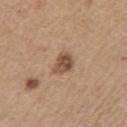<case>
<lighting>white-light</lighting>
<site>left upper arm</site>
<image>
  <source>total-body photography crop</source>
  <field_of_view_mm>15</field_of_view_mm>
</image>
<automated_metrics>
  <area_mm2_approx>5.5</area_mm2_approx>
  <shape_asymmetry>0.3</shape_asymmetry>
  <nevus_likeness_0_100>60</nevus_likeness_0_100>
  <lesion_detection_confidence_0_100>100</lesion_detection_confidence_0_100>
</automated_metrics>
<patient>
  <sex>male</sex>
  <age_approx>65</age_approx>
</patient>
<lesion_size>
  <long_diameter_mm_approx>3.0</long_diameter_mm_approx>
</lesion_size>
</case>